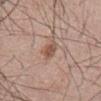Q: Was this lesion biopsied?
A: total-body-photography surveillance lesion; no biopsy
Q: Lesion location?
A: the mid back
Q: What kind of image is this?
A: ~15 mm tile from a whole-body skin photo
Q: Patient demographics?
A: male, in their mid- to late 40s